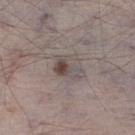Q: Is there a histopathology result?
A: imaged on a skin check; not biopsied
Q: Lesion location?
A: the right lower leg
Q: What is the lesion's diameter?
A: about 4 mm
Q: How was this image acquired?
A: ~15 mm tile from a whole-body skin photo
Q: What are the patient's age and sex?
A: male, in their 70s